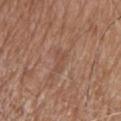biopsy status: imaged on a skin check; not biopsied | location: the head or neck | patient: male, aged approximately 80 | TBP lesion metrics: an area of roughly 3 mm², an outline eccentricity of about 0.9 (0 = round, 1 = elongated), and a symmetry-axis asymmetry near 0.5; a lesion color around L≈49 a*≈20 b*≈28 in CIELAB, a lesion–skin lightness drop of about 6, and a normalized border contrast of about 4.5 | imaging modality: total-body-photography crop, ~15 mm field of view.